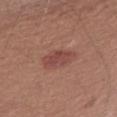follow-up: imaged on a skin check; not biopsied
anatomic site: the arm
subject: male, about 25 years old
illumination: white-light illumination
image-analysis metrics: a footprint of about 7 mm², an eccentricity of roughly 0.85, and a shape-asymmetry score of about 0.2 (0 = symmetric); a lesion color around L≈46 a*≈25 b*≈24 in CIELAB and a normalized lesion–skin contrast near 6.5
diameter: about 4.5 mm
image source: ~15 mm crop, total-body skin-cancer survey